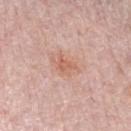biopsy status: no biopsy performed (imaged during a skin exam).